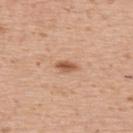<tbp_lesion>
  <biopsy_status>not biopsied; imaged during a skin examination</biopsy_status>
  <patient>
    <sex>male</sex>
    <age_approx>70</age_approx>
  </patient>
  <site>upper back</site>
  <image>
    <source>total-body photography crop</source>
    <field_of_view_mm>15</field_of_view_mm>
  </image>
  <lesion_size>
    <long_diameter_mm_approx>2.5</long_diameter_mm_approx>
  </lesion_size>
  <lighting>white-light</lighting>
</tbp_lesion>